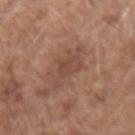No biopsy was performed on this lesion — it was imaged during a full skin examination and was not determined to be concerning.
A male subject, approximately 60 years of age.
Automated image analysis of the tile measured a lesion color around L≈47 a*≈20 b*≈27 in CIELAB and a normalized lesion–skin contrast near 5.5. And it measured a border-irregularity rating of about 7.5/10, a within-lesion color-variation index near 2/10, and peripheral color asymmetry of about 0.5. And it measured a nevus-likeness score of about 0/100 and a detector confidence of about 100 out of 100 that the crop contains a lesion.
This image is a 15 mm lesion crop taken from a total-body photograph.
About 4.5 mm across.
Imaged with white-light lighting.
Located on the right forearm.